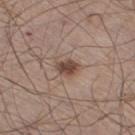<tbp_lesion>
<biopsy_status>not biopsied; imaged during a skin examination</biopsy_status>
<image>
  <source>total-body photography crop</source>
  <field_of_view_mm>15</field_of_view_mm>
</image>
<lesion_size>
  <long_diameter_mm_approx>2.5</long_diameter_mm_approx>
</lesion_size>
<patient>
  <sex>male</sex>
  <age_approx>60</age_approx>
</patient>
<site>right thigh</site>
</tbp_lesion>